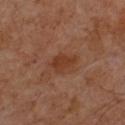biopsy status: imaged on a skin check; not biopsied
site: the chest
subject: male, roughly 60 years of age
automated metrics: an average lesion color of about L≈36 a*≈23 b*≈30 (CIELAB) and about 7 CIELAB-L* units darker than the surrounding skin; a color-variation rating of about 2.5/10 and radial color variation of about 1; lesion-presence confidence of about 100/100
illumination: cross-polarized illumination
image: ~15 mm crop, total-body skin-cancer survey